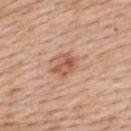workup = total-body-photography surveillance lesion; no biopsy
subject = male, aged approximately 60
illumination = white-light illumination
image source = total-body-photography crop, ~15 mm field of view
anatomic site = the upper back